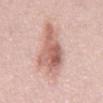Clinical impression:
Part of a total-body skin-imaging series; this lesion was reviewed on a skin check and was not flagged for biopsy.
Context:
Automated tile analysis of the lesion measured an average lesion color of about L≈62 a*≈22 b*≈26 (CIELAB), a lesion–skin lightness drop of about 13, and a lesion-to-skin contrast of about 8 (normalized; higher = more distinct). The analysis additionally found a border-irregularity rating of about 4/10 and peripheral color asymmetry of about 2.5. The analysis additionally found an automated nevus-likeness rating near 60 out of 100 and lesion-presence confidence of about 100/100. Imaged with white-light lighting. A 15 mm close-up tile from a total-body photography series done for melanoma screening. On the abdomen. The patient is a male aged 48 to 52. The lesion's longest dimension is about 8.5 mm.